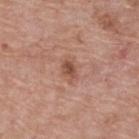Assessment: Captured during whole-body skin photography for melanoma surveillance; the lesion was not biopsied. Acquisition and patient details: A 15 mm crop from a total-body photograph taken for skin-cancer surveillance. Automated image analysis of the tile measured an outline eccentricity of about 0.75 (0 = round, 1 = elongated) and a shape-asymmetry score of about 0.2 (0 = symmetric). The software also gave a nevus-likeness score of about 20/100 and a detector confidence of about 100 out of 100 that the crop contains a lesion. From the back. A female patient, aged 63 to 67. Captured under white-light illumination.